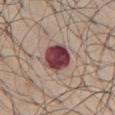No biopsy was performed on this lesion — it was imaged during a full skin examination and was not determined to be concerning.
A male subject aged 68–72.
Cropped from a total-body skin-imaging series; the visible field is about 15 mm.
The lesion is located on the abdomen.
Captured under white-light illumination.
The recorded lesion diameter is about 4 mm.